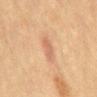No biopsy was performed on this lesion — it was imaged during a full skin examination and was not determined to be concerning. A female subject aged approximately 55. The lesion is located on the mid back. Imaged with cross-polarized lighting. Automated image analysis of the tile measured an average lesion color of about L≈55 a*≈21 b*≈30 (CIELAB). It also reported an automated nevus-likeness rating near 25 out of 100 and a detector confidence of about 100 out of 100 that the crop contains a lesion. A close-up tile cropped from a whole-body skin photograph, about 15 mm across.